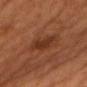Notes:
* workup: imaged on a skin check; not biopsied
* TBP lesion metrics: an area of roughly 7 mm², a shape eccentricity near 0.85, and two-axis asymmetry of about 0.25; a lesion color around L≈34 a*≈25 b*≈32 in CIELAB, about 8 CIELAB-L* units darker than the surrounding skin, and a lesion-to-skin contrast of about 7.5 (normalized; higher = more distinct); a border-irregularity rating of about 3/10, internal color variation of about 2 on a 0–10 scale, and a peripheral color-asymmetry measure near 0.5
* lighting: cross-polarized
* body site: the chest
* diameter: ~4.5 mm (longest diameter)
* patient: male, aged around 40
* acquisition: total-body-photography crop, ~15 mm field of view